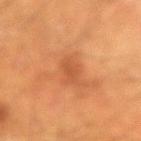Part of a total-body skin-imaging series; this lesion was reviewed on a skin check and was not flagged for biopsy.
The lesion is located on the left forearm.
The tile uses cross-polarized illumination.
Automated image analysis of the tile measured an area of roughly 3.5 mm², an eccentricity of roughly 0.7, and a shape-asymmetry score of about 0.35 (0 = symmetric).
A male patient roughly 60 years of age.
A region of skin cropped from a whole-body photographic capture, roughly 15 mm wide.